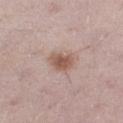Part of a total-body skin-imaging series; this lesion was reviewed on a skin check and was not flagged for biopsy.
The lesion is located on the left thigh.
A female subject, aged 28 to 32.
Captured under white-light illumination.
The total-body-photography lesion software estimated a classifier nevus-likeness of about 90/100 and lesion-presence confidence of about 100/100.
A 15 mm close-up extracted from a 3D total-body photography capture.